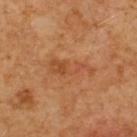Captured during whole-body skin photography for melanoma surveillance; the lesion was not biopsied.
About 5 mm across.
A 15 mm close-up extracted from a 3D total-body photography capture.
An algorithmic analysis of the crop reported a lesion color around L≈44 a*≈23 b*≈35 in CIELAB and about 7 CIELAB-L* units darker than the surrounding skin.
The lesion is located on the upper back.
The subject is a male aged 58–62.